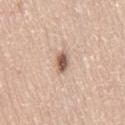Assessment:
The lesion was photographed on a routine skin check and not biopsied; there is no pathology result.
Image and clinical context:
Longest diameter approximately 2.5 mm. Imaged with white-light lighting. A region of skin cropped from a whole-body photographic capture, roughly 15 mm wide. The lesion is on the right thigh. The subject is a female in their mid- to late 40s.